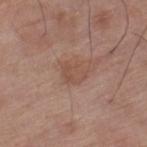workup = total-body-photography surveillance lesion; no biopsy
illumination = white-light illumination
patient = male, aged around 70
location = the right lower leg
size = ≈3 mm
image = total-body-photography crop, ~15 mm field of view